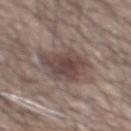This lesion was catalogued during total-body skin photography and was not selected for biopsy.
The lesion's longest dimension is about 5.5 mm.
The subject is a male about 60 years old.
A close-up tile cropped from a whole-body skin photograph, about 15 mm across.
From the mid back.
The tile uses white-light illumination.